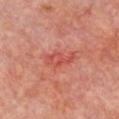{
  "biopsy_status": "not biopsied; imaged during a skin examination",
  "site": "chest",
  "image": {
    "source": "total-body photography crop",
    "field_of_view_mm": 15
  },
  "automated_metrics": {
    "area_mm2_approx": 4.0,
    "eccentricity": 0.9,
    "cielab_L": 52,
    "cielab_a": 36,
    "cielab_b": 31,
    "vs_skin_darker_L": 8.0,
    "vs_skin_contrast_norm": 5.5
  },
  "lighting": "cross-polarized",
  "patient": {
    "sex": "female",
    "age_approx": 50
  }
}